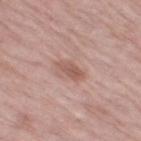notes: no biopsy performed (imaged during a skin exam) | tile lighting: white-light | imaging modality: total-body-photography crop, ~15 mm field of view | patient: female, in their 70s | anatomic site: the right thigh | automated metrics: an area of roughly 4 mm² and a shape-asymmetry score of about 0.25 (0 = symmetric); an average lesion color of about L≈56 a*≈21 b*≈25 (CIELAB) and a lesion–skin lightness drop of about 10; border irregularity of about 3 on a 0–10 scale; a classifier nevus-likeness of about 15/100 and lesion-presence confidence of about 100/100.